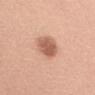  patient:
    sex: female
    age_approx: 35
  site: arm
  image:
    source: total-body photography crop
    field_of_view_mm: 15
  lighting: white-light
  lesion_size:
    long_diameter_mm_approx: 4.0